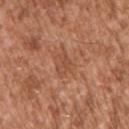workup: imaged on a skin check; not biopsied.